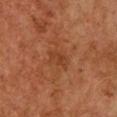Clinical impression: Captured during whole-body skin photography for melanoma surveillance; the lesion was not biopsied. Image and clinical context: Approximately 3 mm at its widest. A female patient, aged around 60. Captured under cross-polarized illumination. Located on the chest. Cropped from a total-body skin-imaging series; the visible field is about 15 mm.